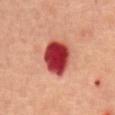No biopsy was performed on this lesion — it was imaged during a full skin examination and was not determined to be concerning. A male subject aged approximately 70. Located on the mid back. A roughly 15 mm field-of-view crop from a total-body skin photograph. An algorithmic analysis of the crop reported an area of roughly 14 mm² and a symmetry-axis asymmetry near 0.15. It also reported a mean CIELAB color near L≈42 a*≈40 b*≈29, about 22 CIELAB-L* units darker than the surrounding skin, and a lesion-to-skin contrast of about 15 (normalized; higher = more distinct). The software also gave a nevus-likeness score of about 0/100 and a detector confidence of about 100 out of 100 that the crop contains a lesion.